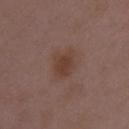automated metrics: an average lesion color of about L≈39 a*≈19 b*≈25 (CIELAB), a lesion–skin lightness drop of about 7, and a normalized lesion–skin contrast near 7; a border-irregularity rating of about 2.5/10, internal color variation of about 2 on a 0–10 scale, and radial color variation of about 0.5; a nevus-likeness score of about 70/100 and lesion-presence confidence of about 100/100
anatomic site: the left upper arm
patient: female, aged around 30
tile lighting: white-light
diameter: about 3.5 mm
image: ~15 mm crop, total-body skin-cancer survey